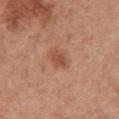  biopsy_status: not biopsied; imaged during a skin examination
  automated_metrics:
    area_mm2_approx: 4.0
    eccentricity: 0.8
    shape_asymmetry: 0.2
    cielab_L: 51
    cielab_a: 24
    cielab_b: 33
    vs_skin_darker_L: 9.0
    vs_skin_contrast_norm: 6.5
    border_irregularity_0_10: 2.0
    color_variation_0_10: 2.0
    peripheral_color_asymmetry: 0.5
    nevus_likeness_0_100: 50
    lesion_detection_confidence_0_100: 100
  lighting: white-light
  patient:
    sex: female
    age_approx: 65
  site: arm
  lesion_size:
    long_diameter_mm_approx: 3.0
  image:
    source: total-body photography crop
    field_of_view_mm: 15